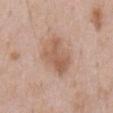The lesion was tiled from a total-body skin photograph and was not biopsied.
On the abdomen.
This is a white-light tile.
A region of skin cropped from a whole-body photographic capture, roughly 15 mm wide.
A male patient, aged around 60.